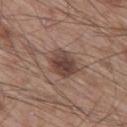  biopsy_status: not biopsied; imaged during a skin examination
  image:
    source: total-body photography crop
    field_of_view_mm: 15
  automated_metrics:
    area_mm2_approx: 9.0
    eccentricity: 0.6
    shape_asymmetry: 0.2
    cielab_L: 43
    cielab_a: 18
    cielab_b: 23
    vs_skin_darker_L: 12.0
  lighting: white-light
  site: leg
  patient:
    sex: male
    age_approx: 60